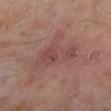Assessment:
No biopsy was performed on this lesion — it was imaged during a full skin examination and was not determined to be concerning.
Acquisition and patient details:
A male subject, roughly 60 years of age. This image is a 15 mm lesion crop taken from a total-body photograph. The total-body-photography lesion software estimated a lesion area of about 11 mm² and an outline eccentricity of about 0.9 (0 = round, 1 = elongated). The software also gave a within-lesion color-variation index near 3/10 and a peripheral color-asymmetry measure near 1. Located on the left lower leg. About 5.5 mm across. Imaged with cross-polarized lighting.